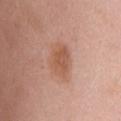Case summary:
– biopsy status — no biopsy performed (imaged during a skin exam)